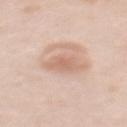{"biopsy_status": "not biopsied; imaged during a skin examination", "image": {"source": "total-body photography crop", "field_of_view_mm": 15}, "patient": {"sex": "female", "age_approx": 50}, "automated_metrics": {"area_mm2_approx": 4.5, "eccentricity": 0.6, "shape_asymmetry": 0.35, "nevus_likeness_0_100": 25, "lesion_detection_confidence_0_100": 100}, "lighting": "white-light", "lesion_size": {"long_diameter_mm_approx": 3.0}, "site": "back"}